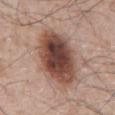Findings:
- notes — imaged on a skin check; not biopsied
- image source — ~15 mm crop, total-body skin-cancer survey
- body site — the abdomen
- patient — male, in their mid- to late 60s